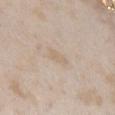The lesion was photographed on a routine skin check and not biopsied; there is no pathology result. The subject is a female approximately 25 years of age. Captured under white-light illumination. An algorithmic analysis of the crop reported an average lesion color of about L≈66 a*≈12 b*≈29 (CIELAB), a lesion–skin lightness drop of about 5, and a normalized lesion–skin contrast near 4.5. It also reported a color-variation rating of about 0.5/10 and a peripheral color-asymmetry measure near 0. Located on the chest. The lesion's longest dimension is about 3 mm. A 15 mm close-up extracted from a 3D total-body photography capture.